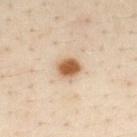Q: Was this lesion biopsied?
A: imaged on a skin check; not biopsied
Q: What lighting was used for the tile?
A: cross-polarized illumination
Q: What is the anatomic site?
A: the chest
Q: What is the imaging modality?
A: ~15 mm tile from a whole-body skin photo
Q: What is the lesion's diameter?
A: ~3 mm (longest diameter)
Q: Who is the patient?
A: male, aged around 30